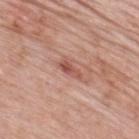Clinical impression: The lesion was photographed on a routine skin check and not biopsied; there is no pathology result. Image and clinical context: A male patient about 60 years old. The tile uses white-light illumination. The lesion is located on the upper back. Cropped from a whole-body photographic skin survey; the tile spans about 15 mm. Measured at roughly 3 mm in maximum diameter.